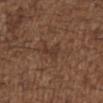| feature | finding |
|---|---|
| notes | no biopsy performed (imaged during a skin exam) |
| body site | the chest |
| image source | 15 mm crop, total-body photography |
| automated metrics | an area of roughly 3 mm² and a shape eccentricity near 0.85; a lesion color around L≈35 a*≈18 b*≈25 in CIELAB, roughly 6 lightness units darker than nearby skin, and a normalized border contrast of about 5.5; a within-lesion color-variation index near 1/10 and peripheral color asymmetry of about 0.5; a nevus-likeness score of about 0/100 and lesion-presence confidence of about 80/100 |
| patient | male, aged 48 to 52 |
| size | ≈2.5 mm |